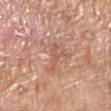automated_metrics:
  cielab_L: 57
  cielab_a: 23
  cielab_b: 30
  vs_skin_contrast_norm: 5.0
  border_irregularity_0_10: 8.5
  peripheral_color_asymmetry: 0.0
patient:
  sex: male
  age_approx: 80
lesion_size:
  long_diameter_mm_approx: 4.0
image:
  source: total-body photography crop
  field_of_view_mm: 15
site: left lower leg